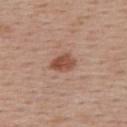This lesion was catalogued during total-body skin photography and was not selected for biopsy.
Imaged with white-light lighting.
The patient is a female roughly 55 years of age.
A roughly 15 mm field-of-view crop from a total-body skin photograph.
Located on the upper back.
The recorded lesion diameter is about 3 mm.